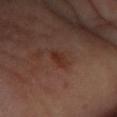Cropped from a whole-body photographic skin survey; the tile spans about 15 mm. The patient is a female aged approximately 50. The tile uses cross-polarized illumination. Measured at roughly 3 mm in maximum diameter. The lesion is on the left forearm.